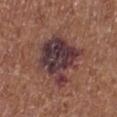Part of a total-body skin-imaging series; this lesion was reviewed on a skin check and was not flagged for biopsy.
Automated tile analysis of the lesion measured border irregularity of about 4 on a 0–10 scale, a color-variation rating of about 7.5/10, and radial color variation of about 2.5.
On the right lower leg.
Longest diameter approximately 6.5 mm.
The patient is a male aged 73 to 77.
A region of skin cropped from a whole-body photographic capture, roughly 15 mm wide.